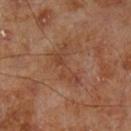| field | value |
|---|---|
| workup | total-body-photography surveillance lesion; no biopsy |